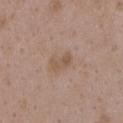patient=female, in their mid- to late 30s | lighting=white-light | image=~15 mm crop, total-body skin-cancer survey | body site=the chest.